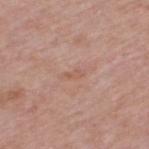The lesion was photographed on a routine skin check and not biopsied; there is no pathology result. A 15 mm crop from a total-body photograph taken for skin-cancer surveillance. A male patient about 55 years old. The lesion is on the upper back. About 2.5 mm across. This is a white-light tile.